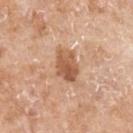  biopsy_status: not biopsied; imaged during a skin examination
  image:
    source: total-body photography crop
    field_of_view_mm: 15
  patient:
    sex: female
    age_approx: 75
  site: left forearm